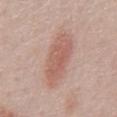patient = male, aged approximately 60; site = the abdomen; diameter = about 6.5 mm; acquisition = 15 mm crop, total-body photography; illumination = white-light.